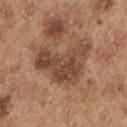{
  "biopsy_status": "not biopsied; imaged during a skin examination",
  "patient": {
    "sex": "male",
    "age_approx": 55
  },
  "image": {
    "source": "total-body photography crop",
    "field_of_view_mm": 15
  },
  "site": "arm",
  "automated_metrics": {
    "lesion_detection_confidence_0_100": 100
  },
  "lighting": "white-light"
}